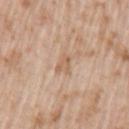No biopsy was performed on this lesion — it was imaged during a full skin examination and was not determined to be concerning.
The lesion is located on the left upper arm.
Cropped from a whole-body photographic skin survey; the tile spans about 15 mm.
The lesion-visualizer software estimated a lesion area of about 3 mm², a shape eccentricity near 0.8, and a shape-asymmetry score of about 0.35 (0 = symmetric). It also reported a color-variation rating of about 1/10 and a peripheral color-asymmetry measure near 0.5. It also reported a classifier nevus-likeness of about 0/100 and a lesion-detection confidence of about 100/100.
Imaged with white-light lighting.
The recorded lesion diameter is about 2.5 mm.
The subject is a male aged 58–62.